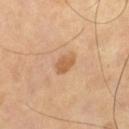<lesion>
  <biopsy_status>not biopsied; imaged during a skin examination</biopsy_status>
  <lesion_size>
    <long_diameter_mm_approx>2.5</long_diameter_mm_approx>
  </lesion_size>
  <automated_metrics>
    <cielab_L>56</cielab_L>
    <cielab_a>21</cielab_a>
    <cielab_b>37</cielab_b>
    <vs_skin_darker_L>10.0</vs_skin_darker_L>
    <vs_skin_contrast_norm>7.5</vs_skin_contrast_norm>
    <border_irregularity_0_10>3.0</border_irregularity_0_10>
    <color_variation_0_10>1.0</color_variation_0_10>
  </automated_metrics>
  <image>
    <source>total-body photography crop</source>
    <field_of_view_mm>15</field_of_view_mm>
  </image>
  <patient>
    <sex>male</sex>
    <age_approx>65</age_approx>
  </patient>
  <site>abdomen</site>
</lesion>